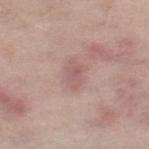Assessment:
Part of a total-body skin-imaging series; this lesion was reviewed on a skin check and was not flagged for biopsy.
Context:
The patient is a male aged 63 to 67. Cropped from a total-body skin-imaging series; the visible field is about 15 mm. About 3 mm across. The tile uses white-light illumination. On the right lower leg.